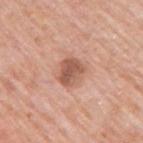The recorded lesion diameter is about 3.5 mm. Captured under white-light illumination. Cropped from a whole-body photographic skin survey; the tile spans about 15 mm. The subject is a male aged around 80. Automated image analysis of the tile measured an area of roughly 7.5 mm², a shape eccentricity near 0.5, and two-axis asymmetry of about 0.2. From the right upper arm.